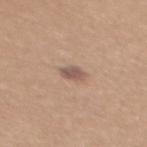- notes — catalogued during a skin exam; not biopsied
- image-analysis metrics — a lesion area of about 3.5 mm² and a shape eccentricity near 0.75; a border-irregularity rating of about 2.5/10 and a within-lesion color-variation index near 2/10
- location — the mid back
- image source — total-body-photography crop, ~15 mm field of view
- diameter — ≈2.5 mm
- tile lighting — white-light illumination
- patient — female, about 40 years old